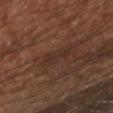Impression:
Recorded during total-body skin imaging; not selected for excision or biopsy.
Image and clinical context:
On the left arm. A male patient in their mid- to late 60s. This image is a 15 mm lesion crop taken from a total-body photograph.